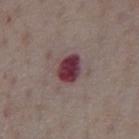Q: Patient demographics?
A: male, aged around 75
Q: What is the anatomic site?
A: the abdomen
Q: What is the imaging modality?
A: ~15 mm crop, total-body skin-cancer survey
Q: Illumination type?
A: white-light illumination
Q: Lesion size?
A: ≈3.5 mm